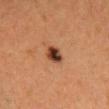Notes:
- workup — total-body-photography surveillance lesion; no biopsy
- size — ≈2.5 mm
- lighting — cross-polarized illumination
- patient — female, approximately 40 years of age
- anatomic site — the head or neck
- acquisition — ~15 mm tile from a whole-body skin photo